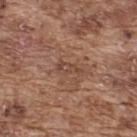Assessment:
Imaged during a routine full-body skin examination; the lesion was not biopsied and no histopathology is available.
Background:
The lesion's longest dimension is about 3 mm. This is a white-light tile. A lesion tile, about 15 mm wide, cut from a 3D total-body photograph. Located on the back. The patient is a male about 75 years old. The lesion-visualizer software estimated a border-irregularity rating of about 4/10 and radial color variation of about 1.